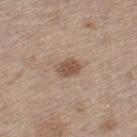Assessment:
Imaged during a routine full-body skin examination; the lesion was not biopsied and no histopathology is available.
Background:
Captured under white-light illumination. Measured at roughly 3 mm in maximum diameter. A male subject aged approximately 70. Automated tile analysis of the lesion measured an area of roughly 5.5 mm², an outline eccentricity of about 0.75 (0 = round, 1 = elongated), and a shape-asymmetry score of about 0.2 (0 = symmetric). The software also gave peripheral color asymmetry of about 0.5. Cropped from a whole-body photographic skin survey; the tile spans about 15 mm. From the left thigh.